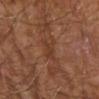Findings:
* follow-up: imaged on a skin check; not biopsied
* subject: male, aged around 70
* image: ~15 mm tile from a whole-body skin photo
* location: the left forearm